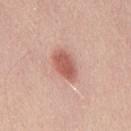A male patient, approximately 40 years of age.
Automated tile analysis of the lesion measured about 13 CIELAB-L* units darker than the surrounding skin and a lesion-to-skin contrast of about 8 (normalized; higher = more distinct). And it measured a border-irregularity rating of about 1.5/10 and a peripheral color-asymmetry measure near 1. The software also gave lesion-presence confidence of about 100/100.
About 4 mm across.
From the mid back.
A 15 mm crop from a total-body photograph taken for skin-cancer surveillance.